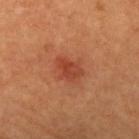Assessment: Imaged during a routine full-body skin examination; the lesion was not biopsied and no histopathology is available. Context: The lesion is on the left upper arm. This image is a 15 mm lesion crop taken from a total-body photograph. The subject is a female about 60 years old.